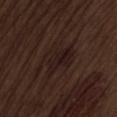Background:
The tile uses white-light illumination. A male patient, in their 70s. Located on the lower back. A 15 mm close-up tile from a total-body photography series done for melanoma screening.